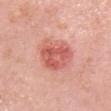Impression:
No biopsy was performed on this lesion — it was imaged during a full skin examination and was not determined to be concerning.
Acquisition and patient details:
A 15 mm close-up tile from a total-body photography series done for melanoma screening. From the head or neck. Imaged with white-light lighting. Measured at roughly 4.5 mm in maximum diameter. A female patient, approximately 60 years of age.